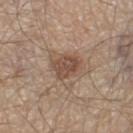| field | value |
|---|---|
| follow-up | no biopsy performed (imaged during a skin exam) |
| image source | ~15 mm tile from a whole-body skin photo |
| body site | the left thigh |
| patient | male, about 60 years old |